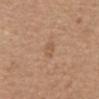Clinical impression: This lesion was catalogued during total-body skin photography and was not selected for biopsy. Context: Imaged with white-light lighting. Cropped from a whole-body photographic skin survey; the tile spans about 15 mm. A female patient, in their mid- to late 70s. Located on the abdomen.